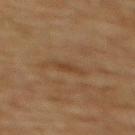<case>
<patient>
  <sex>female</sex>
  <age_approx>55</age_approx>
</patient>
<site>upper back</site>
<image>
  <source>total-body photography crop</source>
  <field_of_view_mm>15</field_of_view_mm>
</image>
<automated_metrics>
  <cielab_L>36</cielab_L>
  <cielab_a>16</cielab_a>
  <cielab_b>28</cielab_b>
  <vs_skin_darker_L>5.0</vs_skin_darker_L>
  <vs_skin_contrast_norm>5.0</vs_skin_contrast_norm>
  <color_variation_0_10>2.0</color_variation_0_10>
  <peripheral_color_asymmetry>0.5</peripheral_color_asymmetry>
</automated_metrics>
<lighting>cross-polarized</lighting>
<lesion_size>
  <long_diameter_mm_approx>3.0</long_diameter_mm_approx>
</lesion_size>
</case>